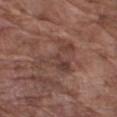<tbp_lesion>
<biopsy_status>not biopsied; imaged during a skin examination</biopsy_status>
<lighting>white-light</lighting>
<patient>
  <sex>male</sex>
  <age_approx>75</age_approx>
</patient>
<site>right upper arm</site>
<automated_metrics>
  <cielab_L>41</cielab_L>
  <cielab_a>19</cielab_a>
  <cielab_b>23</cielab_b>
  <vs_skin_darker_L>7.0</vs_skin_darker_L>
  <vs_skin_contrast_norm>5.5</vs_skin_contrast_norm>
  <border_irregularity_0_10>8.0</border_irregularity_0_10>
  <color_variation_0_10>5.5</color_variation_0_10>
  <peripheral_color_asymmetry>2.0</peripheral_color_asymmetry>
</automated_metrics>
<image>
  <source>total-body photography crop</source>
  <field_of_view_mm>15</field_of_view_mm>
</image>
<lesion_size>
  <long_diameter_mm_approx>6.0</long_diameter_mm_approx>
</lesion_size>
</tbp_lesion>